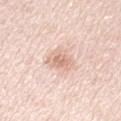subject: female, aged 63–67
image: ~15 mm crop, total-body skin-cancer survey
TBP lesion metrics: border irregularity of about 2.5 on a 0–10 scale and a within-lesion color-variation index near 3/10; a nevus-likeness score of about 5/100 and a lesion-detection confidence of about 100/100
tile lighting: white-light
diameter: ≈3 mm
site: the left upper arm